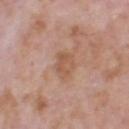notes = catalogued during a skin exam; not biopsied | subject = male, approximately 70 years of age | image source = ~15 mm tile from a whole-body skin photo | lesion size = ~3 mm (longest diameter) | body site = the head or neck.